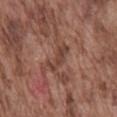patient:
  sex: male
  age_approx: 75
automated_metrics:
  area_mm2_approx: 5.0
  eccentricity: 0.95
  cielab_L: 41
  cielab_a: 21
  cielab_b: 25
  vs_skin_darker_L: 8.0
  vs_skin_contrast_norm: 6.5
  border_irregularity_0_10: 5.5
  color_variation_0_10: 1.0
  peripheral_color_asymmetry: 0.0
  lesion_detection_confidence_0_100: 70
lighting: white-light
image:
  source: total-body photography crop
  field_of_view_mm: 15
site: mid back